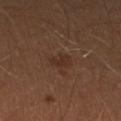No biopsy was performed on this lesion — it was imaged during a full skin examination and was not determined to be concerning.
The patient is a male about 40 years old.
A 15 mm crop from a total-body photograph taken for skin-cancer surveillance.
The total-body-photography lesion software estimated an area of roughly 4 mm².
Captured under cross-polarized illumination.
The lesion is located on the left lower leg.